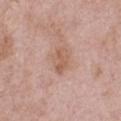This lesion was catalogued during total-body skin photography and was not selected for biopsy. A close-up tile cropped from a whole-body skin photograph, about 15 mm across. A male subject, approximately 50 years of age. On the chest.